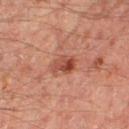| field | value |
|---|---|
| follow-up | imaged on a skin check; not biopsied |
| image-analysis metrics | a footprint of about 5.5 mm², an eccentricity of roughly 0.65, and two-axis asymmetry of about 0.3; a color-variation rating of about 8/10; a classifier nevus-likeness of about 75/100 and a detector confidence of about 100 out of 100 that the crop contains a lesion |
| acquisition | ~15 mm tile from a whole-body skin photo |
| lesion size | about 3 mm |
| illumination | cross-polarized illumination |
| anatomic site | the right thigh |
| patient | male, in their mid-40s |